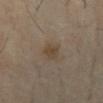follow-up: imaged on a skin check; not biopsied
imaging modality: ~15 mm tile from a whole-body skin photo
tile lighting: cross-polarized illumination
lesion diameter: about 2.5 mm
anatomic site: the front of the torso
subject: male, in their mid-60s
automated metrics: internal color variation of about 2 on a 0–10 scale